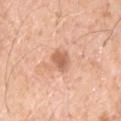{"biopsy_status": "not biopsied; imaged during a skin examination", "lighting": "white-light", "site": "upper back", "automated_metrics": {"cielab_L": 62, "cielab_a": 23, "cielab_b": 33, "vs_skin_darker_L": 12.0, "vs_skin_contrast_norm": 7.0}, "patient": {"sex": "male", "age_approx": 70}, "image": {"source": "total-body photography crop", "field_of_view_mm": 15}, "lesion_size": {"long_diameter_mm_approx": 2.5}}